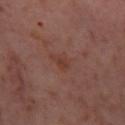Context:
A 15 mm crop from a total-body photograph taken for skin-cancer surveillance. A female patient, aged 53 to 57. On the right thigh.